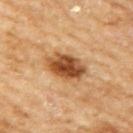Notes:
* lesion size · about 4.5 mm
* patient · female, in their 60s
* lighting · cross-polarized illumination
* location · the right upper arm
* acquisition · ~15 mm tile from a whole-body skin photo
* automated metrics · an area of roughly 12 mm² and two-axis asymmetry of about 0.2; an average lesion color of about L≈50 a*≈23 b*≈39 (CIELAB) and a lesion-to-skin contrast of about 11.5 (normalized; higher = more distinct); a border-irregularity index near 2/10 and peripheral color asymmetry of about 2; an automated nevus-likeness rating near 95 out of 100 and a detector confidence of about 100 out of 100 that the crop contains a lesion